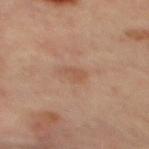workup: no biopsy performed (imaged during a skin exam) | body site: the mid back | imaging modality: ~15 mm crop, total-body skin-cancer survey | subject: female, aged 43–47 | automated lesion analysis: a footprint of about 3.5 mm², an eccentricity of roughly 0.75, and a symmetry-axis asymmetry near 0.35; border irregularity of about 3.5 on a 0–10 scale, a color-variation rating of about 1/10, and radial color variation of about 0.5; a classifier nevus-likeness of about 0/100 and a detector confidence of about 100 out of 100 that the crop contains a lesion | tile lighting: cross-polarized illumination | diameter: ≈2.5 mm.